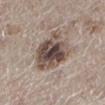{
  "site": "left lower leg",
  "lesion_size": {
    "long_diameter_mm_approx": 5.5
  },
  "image": {
    "source": "total-body photography crop",
    "field_of_view_mm": 15
  },
  "lighting": "white-light",
  "patient": {
    "sex": "female",
    "age_approx": 50
  }
}